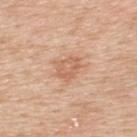workup: no biopsy performed (imaged during a skin exam)
location: the back
patient: male, aged approximately 50
lighting: white-light illumination
imaging modality: total-body-photography crop, ~15 mm field of view
size: ~3 mm (longest diameter)